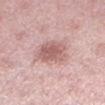Clinical impression: The lesion was photographed on a routine skin check and not biopsied; there is no pathology result. Acquisition and patient details: Located on the left lower leg. The patient is a female aged 38 to 42. The lesion's longest dimension is about 4.5 mm. Cropped from a whole-body photographic skin survey; the tile spans about 15 mm. The lesion-visualizer software estimated a shape eccentricity near 0.7 and a shape-asymmetry score of about 0.2 (0 = symmetric). The software also gave a lesion color around L≈60 a*≈22 b*≈22 in CIELAB and a lesion–skin lightness drop of about 11. And it measured a border-irregularity index near 2/10 and peripheral color asymmetry of about 1.